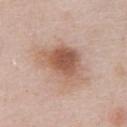Cropped from a whole-body photographic skin survey; the tile spans about 15 mm. Captured under white-light illumination. The subject is a male aged around 55. Longest diameter approximately 6.5 mm. Located on the abdomen.